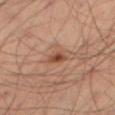Imaged during a routine full-body skin examination; the lesion was not biopsied and no histopathology is available. Cropped from a total-body skin-imaging series; the visible field is about 15 mm. A male subject aged 53–57. Located on the right thigh. Approximately 2.5 mm at its widest.